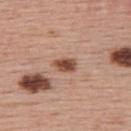Notes:
- workup · total-body-photography surveillance lesion; no biopsy
- image source · 15 mm crop, total-body photography
- location · the upper back
- subject · female, in their mid- to late 50s